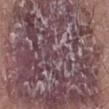<record>
<biopsy_status>not biopsied; imaged during a skin examination</biopsy_status>
<automated_metrics>
  <cielab_L>45</cielab_L>
  <cielab_a>18</cielab_a>
  <cielab_b>13</cielab_b>
  <vs_skin_contrast_norm>9.0</vs_skin_contrast_norm>
  <peripheral_color_asymmetry>3.5</peripheral_color_asymmetry>
</automated_metrics>
<patient>
  <sex>female</sex>
  <age_approx>75</age_approx>
</patient>
<image>
  <source>total-body photography crop</source>
  <field_of_view_mm>15</field_of_view_mm>
</image>
<site>right lower leg</site>
<lesion_size>
  <long_diameter_mm_approx>15.5</long_diameter_mm_approx>
</lesion_size>
<lighting>white-light</lighting>
</record>